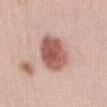{
  "biopsy_status": "not biopsied; imaged during a skin examination",
  "automated_metrics": {
    "eccentricity": 0.6,
    "shape_asymmetry": 0.15,
    "border_irregularity_0_10": 1.5,
    "color_variation_0_10": 5.0,
    "peripheral_color_asymmetry": 2.0
  },
  "lesion_size": {
    "long_diameter_mm_approx": 5.0
  },
  "patient": {
    "sex": "female",
    "age_approx": 40
  },
  "lighting": "white-light",
  "image": {
    "source": "total-body photography crop",
    "field_of_view_mm": 15
  },
  "site": "front of the torso"
}